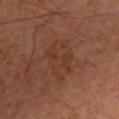The lesion was photographed on a routine skin check and not biopsied; there is no pathology result. Cropped from a whole-body photographic skin survey; the tile spans about 15 mm. Longest diameter approximately 5.5 mm. The lesion-visualizer software estimated an area of roughly 13 mm², a shape eccentricity near 0.8, and two-axis asymmetry of about 0.25. And it measured a mean CIELAB color near L≈35 a*≈20 b*≈28 and a normalized border contrast of about 5. And it measured a nevus-likeness score of about 0/100 and a lesion-detection confidence of about 100/100. The lesion is on the left upper arm. Imaged with cross-polarized lighting. A male patient aged approximately 65.